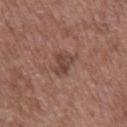biopsy status=imaged on a skin check; not biopsied
patient=male, aged around 50
image-analysis metrics=a mean CIELAB color near L≈43 a*≈20 b*≈24; a border-irregularity rating of about 3.5/10 and a peripheral color-asymmetry measure near 1; a classifier nevus-likeness of about 0/100 and a lesion-detection confidence of about 100/100
illumination=white-light illumination
lesion size=about 2.5 mm
site=the mid back
acquisition=~15 mm tile from a whole-body skin photo